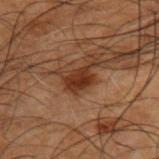Image and clinical context: This is a cross-polarized tile. The subject is a male about 50 years old. The lesion is located on the upper back. Cropped from a total-body skin-imaging series; the visible field is about 15 mm.